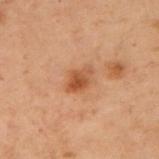Part of a total-body skin-imaging series; this lesion was reviewed on a skin check and was not flagged for biopsy. A 15 mm close-up extracted from a 3D total-body photography capture. Measured at roughly 3 mm in maximum diameter. A female subject, about 55 years old. The lesion is on the left upper arm. Imaged with cross-polarized lighting.